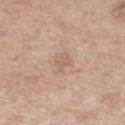workup: imaged on a skin check; not biopsied
automated lesion analysis: an outline eccentricity of about 0.75 (0 = round, 1 = elongated) and a symmetry-axis asymmetry near 0.3; a border-irregularity index near 3/10; a nevus-likeness score of about 0/100 and a lesion-detection confidence of about 100/100
anatomic site: the right thigh
image source: ~15 mm crop, total-body skin-cancer survey
illumination: white-light
patient: female, aged 53 to 57